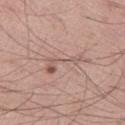The lesion was tiled from a total-body skin photograph and was not biopsied.
The patient is a male aged 38–42.
This is a white-light tile.
A lesion tile, about 15 mm wide, cut from a 3D total-body photograph.
About 5 mm across.
Automated image analysis of the tile measured a footprint of about 7 mm², an eccentricity of roughly 0.95, and two-axis asymmetry of about 0.65. And it measured a border-irregularity index near 9/10 and radial color variation of about 1.5.
Located on the left thigh.